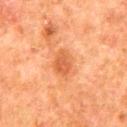Q: Was this lesion biopsied?
A: imaged on a skin check; not biopsied
Q: Where on the body is the lesion?
A: the mid back
Q: What kind of image is this?
A: total-body-photography crop, ~15 mm field of view
Q: Who is the patient?
A: male, aged 78 to 82